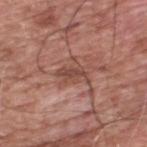The tile uses white-light illumination. The lesion is located on the upper back. A male patient, aged approximately 60. Longest diameter approximately 3.5 mm. The lesion-visualizer software estimated a footprint of about 6.5 mm², an outline eccentricity of about 0.6 (0 = round, 1 = elongated), and two-axis asymmetry of about 0.4. The software also gave border irregularity of about 5.5 on a 0–10 scale and a color-variation rating of about 2.5/10. And it measured a nevus-likeness score of about 0/100. A close-up tile cropped from a whole-body skin photograph, about 15 mm across.